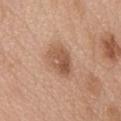Recorded during total-body skin imaging; not selected for excision or biopsy. A lesion tile, about 15 mm wide, cut from a 3D total-body photograph. On the abdomen. The subject is a female in their 60s.